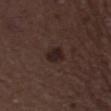<record>
  <automated_metrics>
    <area_mm2_approx>4.5</area_mm2_approx>
    <eccentricity>0.65</eccentricity>
    <shape_asymmetry>0.25</shape_asymmetry>
  </automated_metrics>
  <patient>
    <sex>male</sex>
    <age_approx>50</age_approx>
  </patient>
  <image>
    <source>total-body photography crop</source>
    <field_of_view_mm>15</field_of_view_mm>
  </image>
  <site>right thigh</site>
  <lesion_size>
    <long_diameter_mm_approx>2.5</long_diameter_mm_approx>
  </lesion_size>
</record>